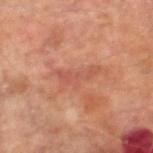biopsy status: catalogued during a skin exam; not biopsied | location: the right lower leg | patient: male, about 65 years old | tile lighting: cross-polarized illumination | TBP lesion metrics: a footprint of about 7.5 mm², a shape eccentricity near 0.9, and a shape-asymmetry score of about 0.55 (0 = symmetric); a peripheral color-asymmetry measure near 1; a classifier nevus-likeness of about 0/100 and lesion-presence confidence of about 95/100 | imaging modality: ~15 mm tile from a whole-body skin photo | diameter: ~5 mm (longest diameter).